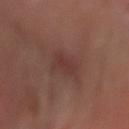Impression:
No biopsy was performed on this lesion — it was imaged during a full skin examination and was not determined to be concerning.
Context:
A male patient, in their mid- to late 60s. A region of skin cropped from a whole-body photographic capture, roughly 15 mm wide. Located on the right forearm. An algorithmic analysis of the crop reported a lesion color around L≈35 a*≈21 b*≈22 in CIELAB, roughly 6 lightness units darker than nearby skin, and a lesion-to-skin contrast of about 5.5 (normalized; higher = more distinct). The software also gave a border-irregularity index near 3/10 and a peripheral color-asymmetry measure near 0.5. And it measured a nevus-likeness score of about 20/100 and lesion-presence confidence of about 95/100. The lesion's longest dimension is about 3 mm.